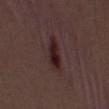Assessment:
The lesion was tiled from a total-body skin photograph and was not biopsied.
Background:
This is a white-light tile. A male patient approximately 55 years of age. Approximately 5 mm at its widest. A 15 mm close-up tile from a total-body photography series done for melanoma screening. On the mid back.